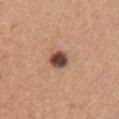biopsy status: no biopsy performed (imaged during a skin exam)
acquisition: total-body-photography crop, ~15 mm field of view
illumination: white-light
site: the front of the torso
subject: female, aged around 25
automated metrics: a lesion area of about 5 mm², an outline eccentricity of about 0.55 (0 = round, 1 = elongated), and a shape-asymmetry score of about 0.15 (0 = symmetric); border irregularity of about 1.5 on a 0–10 scale, a color-variation rating of about 6/10, and a peripheral color-asymmetry measure near 2